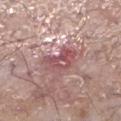No biopsy was performed on this lesion — it was imaged during a full skin examination and was not determined to be concerning. The subject is a male approximately 65 years of age. About 4.5 mm across. The lesion-visualizer software estimated a lesion area of about 8.5 mm², a shape eccentricity near 0.85, and two-axis asymmetry of about 0.4. And it measured a lesion color around L≈52 a*≈26 b*≈19 in CIELAB. The analysis additionally found a border-irregularity index near 4.5/10, a color-variation rating of about 8/10, and a peripheral color-asymmetry measure near 3.5. The software also gave a lesion-detection confidence of about 55/100. A 15 mm close-up tile from a total-body photography series done for melanoma screening. On the left lower leg.